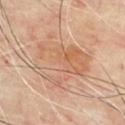follow-up: no biopsy performed (imaged during a skin exam); patient: male, aged around 65; imaging modality: total-body-photography crop, ~15 mm field of view; site: the chest.